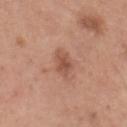Imaged during a routine full-body skin examination; the lesion was not biopsied and no histopathology is available.
Located on the head or neck.
A male patient, aged 33 to 37.
This is a white-light tile.
A close-up tile cropped from a whole-body skin photograph, about 15 mm across.